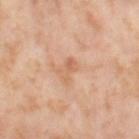The lesion was photographed on a routine skin check and not biopsied; there is no pathology result. Located on the right thigh. The lesion's longest dimension is about 3 mm. A 15 mm close-up tile from a total-body photography series done for melanoma screening. This is a cross-polarized tile. A female subject approximately 55 years of age. The lesion-visualizer software estimated a lesion color around L≈63 a*≈23 b*≈34 in CIELAB, roughly 8 lightness units darker than nearby skin, and a normalized lesion–skin contrast near 5.